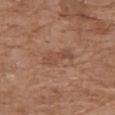biopsy status: no biopsy performed (imaged during a skin exam).